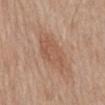The lesion was photographed on a routine skin check and not biopsied; there is no pathology result.
Automated tile analysis of the lesion measured a lesion area of about 14 mm², an eccentricity of roughly 0.8, and two-axis asymmetry of about 0.15. It also reported roughly 7 lightness units darker than nearby skin and a lesion-to-skin contrast of about 5.5 (normalized; higher = more distinct). It also reported border irregularity of about 2 on a 0–10 scale, a within-lesion color-variation index near 2.5/10, and peripheral color asymmetry of about 1.
Cropped from a whole-body photographic skin survey; the tile spans about 15 mm.
This is a white-light tile.
A male subject aged 78–82.
The lesion is on the abdomen.